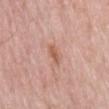The lesion was tiled from a total-body skin photograph and was not biopsied. The subject is a male in their mid-70s. Longest diameter approximately 2.5 mm. The tile uses white-light illumination. Cropped from a whole-body photographic skin survey; the tile spans about 15 mm. The lesion is located on the mid back. The lesion-visualizer software estimated a shape eccentricity near 0.85 and a symmetry-axis asymmetry near 0.25. The analysis additionally found a lesion color around L≈58 a*≈23 b*≈30 in CIELAB, a lesion–skin lightness drop of about 9, and a normalized lesion–skin contrast near 7. The analysis additionally found a nevus-likeness score of about 0/100 and a detector confidence of about 100 out of 100 that the crop contains a lesion.